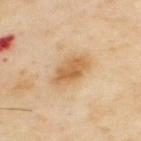biopsy status: imaged on a skin check; not biopsied.